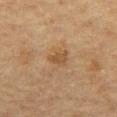The lesion was photographed on a routine skin check and not biopsied; there is no pathology result.
A 15 mm close-up tile from a total-body photography series done for melanoma screening.
The subject is a male approximately 75 years of age.
The lesion is on the abdomen.